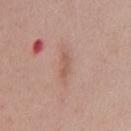Impression:
No biopsy was performed on this lesion — it was imaged during a full skin examination and was not determined to be concerning.
Clinical summary:
A 15 mm close-up tile from a total-body photography series done for melanoma screening. A female subject aged approximately 40. The total-body-photography lesion software estimated a footprint of about 2.5 mm², an eccentricity of roughly 0.95, and two-axis asymmetry of about 0.55. And it measured an average lesion color of about L≈56 a*≈22 b*≈27 (CIELAB), roughly 8 lightness units darker than nearby skin, and a normalized border contrast of about 5.5. And it measured an automated nevus-likeness rating near 0 out of 100 and lesion-presence confidence of about 100/100. Located on the chest. This is a white-light tile. Measured at roughly 2.5 mm in maximum diameter.